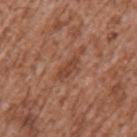<record>
  <biopsy_status>not biopsied; imaged during a skin examination</biopsy_status>
  <lesion_size>
    <long_diameter_mm_approx>3.0</long_diameter_mm_approx>
  </lesion_size>
  <patient>
    <sex>male</sex>
    <age_approx>45</age_approx>
  </patient>
  <site>left upper arm</site>
  <image>
    <source>total-body photography crop</source>
    <field_of_view_mm>15</field_of_view_mm>
  </image>
</record>